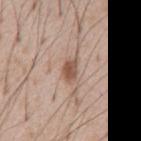Assessment:
Part of a total-body skin-imaging series; this lesion was reviewed on a skin check and was not flagged for biopsy.
Image and clinical context:
The lesion is located on the abdomen. A male patient, approximately 55 years of age. Measured at roughly 3 mm in maximum diameter. Imaged with white-light lighting. A region of skin cropped from a whole-body photographic capture, roughly 15 mm wide.